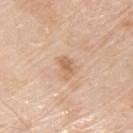| key | value |
|---|---|
| notes | no biopsy performed (imaged during a skin exam) |
| automated lesion analysis | internal color variation of about 1 on a 0–10 scale and a peripheral color-asymmetry measure near 0.5 |
| tile lighting | white-light |
| acquisition | ~15 mm crop, total-body skin-cancer survey |
| patient | male, approximately 80 years of age |
| diameter | ≈2.5 mm |
| body site | the upper back |